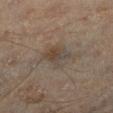– follow-up · imaged on a skin check; not biopsied
– subject · male, roughly 45 years of age
– lesion size · about 3 mm
– illumination · cross-polarized illumination
– body site · the left lower leg
– image source · 15 mm crop, total-body photography